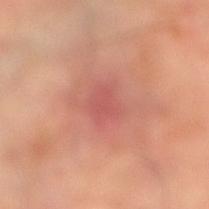<case>
  <biopsy_status>not biopsied; imaged during a skin examination</biopsy_status>
  <patient>
    <sex>female</sex>
    <age_approx>60</age_approx>
  </patient>
  <image>
    <source>total-body photography crop</source>
    <field_of_view_mm>15</field_of_view_mm>
  </image>
  <site>left leg</site>
</case>